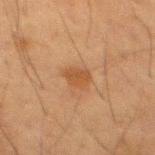No biopsy was performed on this lesion — it was imaged during a full skin examination and was not determined to be concerning. About 2.5 mm across. A lesion tile, about 15 mm wide, cut from a 3D total-body photograph. From the mid back. This is a cross-polarized tile. The total-body-photography lesion software estimated a border-irregularity rating of about 2.5/10, a color-variation rating of about 1.5/10, and peripheral color asymmetry of about 0.5. The software also gave a detector confidence of about 100 out of 100 that the crop contains a lesion. A male patient, approximately 35 years of age.